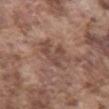| key | value |
|---|---|
| biopsy status | catalogued during a skin exam; not biopsied |
| anatomic site | the chest |
| image | total-body-photography crop, ~15 mm field of view |
| tile lighting | white-light |
| subject | male, approximately 75 years of age |